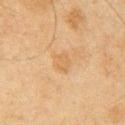Recorded during total-body skin imaging; not selected for excision or biopsy.
Measured at roughly 2.5 mm in maximum diameter.
Located on the right upper arm.
This image is a 15 mm lesion crop taken from a total-body photograph.
A male patient about 65 years old.
Automated tile analysis of the lesion measured a lesion area of about 4 mm², an outline eccentricity of about 0.6 (0 = round, 1 = elongated), and a shape-asymmetry score of about 0.3 (0 = symmetric). It also reported a nevus-likeness score of about 0/100.
This is a cross-polarized tile.